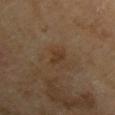Notes:
– biopsy status: catalogued during a skin exam; not biopsied
– patient: male, approximately 70 years of age
– image: 15 mm crop, total-body photography
– body site: the left upper arm